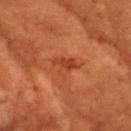This lesion was catalogued during total-body skin photography and was not selected for biopsy. The total-body-photography lesion software estimated about 6 CIELAB-L* units darker than the surrounding skin and a lesion-to-skin contrast of about 6 (normalized; higher = more distinct). It also reported border irregularity of about 5.5 on a 0–10 scale, a color-variation rating of about 1.5/10, and radial color variation of about 0.5. And it measured lesion-presence confidence of about 100/100. The lesion is located on the back. Cropped from a whole-body photographic skin survey; the tile spans about 15 mm. Captured under cross-polarized illumination. A female patient, aged approximately 80. The lesion's longest dimension is about 3 mm.